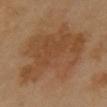The lesion was photographed on a routine skin check and not biopsied; there is no pathology result. An algorithmic analysis of the crop reported an area of roughly 48 mm², a shape eccentricity near 0.7, and a symmetry-axis asymmetry near 0.4. And it measured a mean CIELAB color near L≈43 a*≈19 b*≈32 and a lesion–skin lightness drop of about 7. The analysis additionally found a classifier nevus-likeness of about 30/100 and a detector confidence of about 100 out of 100 that the crop contains a lesion. A close-up tile cropped from a whole-body skin photograph, about 15 mm across. The subject is a female approximately 35 years of age. About 10 mm across. On the front of the torso.